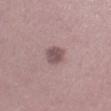lesion diameter=~2.5 mm (longest diameter)
subject=male, aged 43 to 47
location=the right lower leg
acquisition=15 mm crop, total-body photography
image-analysis metrics=a border-irregularity index near 1.5/10, a color-variation rating of about 2.5/10, and a peripheral color-asymmetry measure near 1; a nevus-likeness score of about 10/100 and a lesion-detection confidence of about 100/100